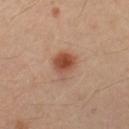This lesion was catalogued during total-body skin photography and was not selected for biopsy. The patient is a female approximately 40 years of age. A 15 mm crop from a total-body photograph taken for skin-cancer surveillance. On the right leg.